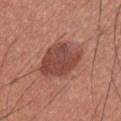Part of a total-body skin-imaging series; this lesion was reviewed on a skin check and was not flagged for biopsy. From the chest. The tile uses white-light illumination. A close-up tile cropped from a whole-body skin photograph, about 15 mm across. Approximately 5 mm at its widest. A male subject aged around 35.